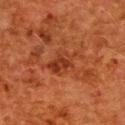workup: total-body-photography surveillance lesion; no biopsy
image-analysis metrics: a footprint of about 7.5 mm², an outline eccentricity of about 0.75 (0 = round, 1 = elongated), and a symmetry-axis asymmetry near 0.35
imaging modality: total-body-photography crop, ~15 mm field of view
diameter: about 4.5 mm
tile lighting: cross-polarized illumination
subject: female, aged 48–52
site: the upper back